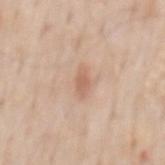No biopsy was performed on this lesion — it was imaged during a full skin examination and was not determined to be concerning. The lesion is located on the mid back. This image is a 15 mm lesion crop taken from a total-body photograph. The tile uses white-light illumination. A male subject, roughly 60 years of age.